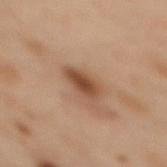Recorded during total-body skin imaging; not selected for excision or biopsy. The lesion is located on the upper back. A lesion tile, about 15 mm wide, cut from a 3D total-body photograph. The lesion's longest dimension is about 3 mm. The tile uses cross-polarized illumination. A female subject in their mid- to late 50s.